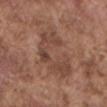Q: Was a biopsy performed?
A: no biopsy performed (imaged during a skin exam)
Q: Automated lesion metrics?
A: an area of roughly 20 mm², an eccentricity of roughly 0.85, and a shape-asymmetry score of about 0.4 (0 = symmetric); a mean CIELAB color near L≈45 a*≈19 b*≈26, a lesion–skin lightness drop of about 7, and a lesion-to-skin contrast of about 6 (normalized; higher = more distinct); a detector confidence of about 55 out of 100 that the crop contains a lesion
Q: What is the lesion's diameter?
A: ≈7.5 mm
Q: What is the imaging modality?
A: ~15 mm tile from a whole-body skin photo
Q: Who is the patient?
A: male, roughly 75 years of age
Q: Where on the body is the lesion?
A: the mid back